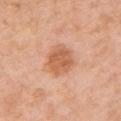This lesion was catalogued during total-body skin photography and was not selected for biopsy.
A female subject aged 53 to 57.
Captured under white-light illumination.
Located on the left upper arm.
Longest diameter approximately 4 mm.
A 15 mm close-up extracted from a 3D total-body photography capture.